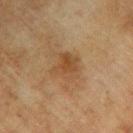Impression:
Imaged during a routine full-body skin examination; the lesion was not biopsied and no histopathology is available.
Background:
On the right forearm. The subject is a male about 75 years old. A close-up tile cropped from a whole-body skin photograph, about 15 mm across. Captured under cross-polarized illumination. The lesion-visualizer software estimated two-axis asymmetry of about 0.3. And it measured roughly 7 lightness units darker than nearby skin and a normalized border contrast of about 6.5. The analysis additionally found border irregularity of about 3.5 on a 0–10 scale and peripheral color asymmetry of about 1. Longest diameter approximately 4 mm.